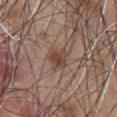The lesion was photographed on a routine skin check and not biopsied; there is no pathology result.
A male patient, aged 43–47.
From the chest.
The lesion's longest dimension is about 3 mm.
An algorithmic analysis of the crop reported a border-irregularity index near 3/10, a within-lesion color-variation index near 5/10, and radial color variation of about 2. The software also gave a nevus-likeness score of about 65/100 and lesion-presence confidence of about 100/100.
The tile uses white-light illumination.
Cropped from a whole-body photographic skin survey; the tile spans about 15 mm.